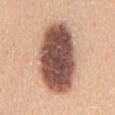biopsy status = imaged on a skin check; not biopsied | subject = male, aged approximately 30 | body site = the mid back | imaging modality = ~15 mm tile from a whole-body skin photo | diameter = ≈9.5 mm | automated lesion analysis = an average lesion color of about L≈52 a*≈20 b*≈26 (CIELAB), roughly 23 lightness units darker than nearby skin, and a normalized lesion–skin contrast near 14.5; border irregularity of about 1.5 on a 0–10 scale, a within-lesion color-variation index near 7/10, and peripheral color asymmetry of about 2; a nevus-likeness score of about 10/100 and a lesion-detection confidence of about 100/100.